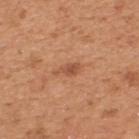Imaged during a routine full-body skin examination; the lesion was not biopsied and no histopathology is available.
The total-body-photography lesion software estimated a mean CIELAB color near L≈53 a*≈25 b*≈35, a lesion–skin lightness drop of about 9, and a lesion-to-skin contrast of about 6.5 (normalized; higher = more distinct). The analysis additionally found a border-irregularity rating of about 3/10, a within-lesion color-variation index near 2/10, and peripheral color asymmetry of about 0.5. The analysis additionally found a classifier nevus-likeness of about 10/100.
The lesion is on the upper back.
This is a white-light tile.
A male subject, aged approximately 55.
A region of skin cropped from a whole-body photographic capture, roughly 15 mm wide.
About 3 mm across.